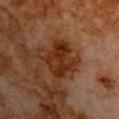The lesion was tiled from a total-body skin photograph and was not biopsied. Approximately 5 mm at its widest. A 15 mm close-up tile from a total-body photography series done for melanoma screening. The tile uses cross-polarized illumination. The lesion-visualizer software estimated a lesion area of about 13 mm², an outline eccentricity of about 0.7 (0 = round, 1 = elongated), and a symmetry-axis asymmetry near 0.2. The lesion is on the chest. A male subject, aged 78–82.